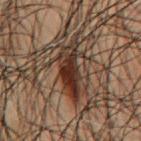Impression:
The lesion was photographed on a routine skin check and not biopsied; there is no pathology result.
Clinical summary:
A male subject, aged approximately 50. The recorded lesion diameter is about 6 mm. A region of skin cropped from a whole-body photographic capture, roughly 15 mm wide. Imaged with cross-polarized lighting. The lesion is located on the mid back.